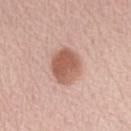Impression:
This lesion was catalogued during total-body skin photography and was not selected for biopsy.
Image and clinical context:
A roughly 15 mm field-of-view crop from a total-body skin photograph. The tile uses white-light illumination. A female subject aged 63 to 67. An algorithmic analysis of the crop reported an area of roughly 12 mm² and an outline eccentricity of about 0.55 (0 = round, 1 = elongated). And it measured a mean CIELAB color near L≈58 a*≈23 b*≈28 and a normalized border contrast of about 9. The software also gave a border-irregularity index near 1/10 and a color-variation rating of about 4/10. And it measured an automated nevus-likeness rating near 95 out of 100 and a detector confidence of about 100 out of 100 that the crop contains a lesion. From the left forearm.